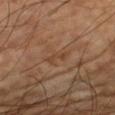<record>
<biopsy_status>not biopsied; imaged during a skin examination</biopsy_status>
<image>
  <source>total-body photography crop</source>
  <field_of_view_mm>15</field_of_view_mm>
</image>
<site>right upper arm</site>
<lesion_size>
  <long_diameter_mm_approx>3.0</long_diameter_mm_approx>
</lesion_size>
<lighting>cross-polarized</lighting>
<patient>
  <sex>male</sex>
  <age_approx>60</age_approx>
</patient>
</record>